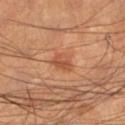This lesion was catalogued during total-body skin photography and was not selected for biopsy. A male subject, roughly 65 years of age. Automated tile analysis of the lesion measured an average lesion color of about L≈47 a*≈25 b*≈33 (CIELAB), about 8 CIELAB-L* units darker than the surrounding skin, and a normalized border contrast of about 6. The analysis additionally found a nevus-likeness score of about 70/100 and a lesion-detection confidence of about 100/100. Measured at roughly 3 mm in maximum diameter. The lesion is located on the right lower leg. Cropped from a total-body skin-imaging series; the visible field is about 15 mm.